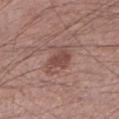biopsy status: no biopsy performed (imaged during a skin exam)
location: the left lower leg
diameter: ≈4 mm
automated metrics: a mean CIELAB color near L≈46 a*≈22 b*≈23, a lesion–skin lightness drop of about 9, and a lesion-to-skin contrast of about 7 (normalized; higher = more distinct); a border-irregularity index near 4.5/10, internal color variation of about 1.5 on a 0–10 scale, and radial color variation of about 0.5; an automated nevus-likeness rating near 20 out of 100 and lesion-presence confidence of about 100/100
patient: male, roughly 55 years of age
image source: ~15 mm tile from a whole-body skin photo